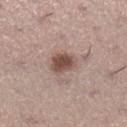This lesion was catalogued during total-body skin photography and was not selected for biopsy. The lesion is located on the right lower leg. A close-up tile cropped from a whole-body skin photograph, about 15 mm across. Captured under white-light illumination. An algorithmic analysis of the crop reported an average lesion color of about L≈49 a*≈18 b*≈23 (CIELAB), a lesion–skin lightness drop of about 14, and a normalized border contrast of about 10. The analysis additionally found a color-variation rating of about 2.5/10. A female patient, about 40 years old.